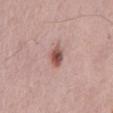Findings:
* follow-up · catalogued during a skin exam; not biopsied
* image · total-body-photography crop, ~15 mm field of view
* tile lighting · white-light
* site · the mid back
* subject · male, aged 63 to 67